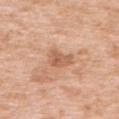  biopsy_status: not biopsied; imaged during a skin examination
  lighting: white-light
  patient:
    sex: female
    age_approx: 40
  lesion_size:
    long_diameter_mm_approx: 3.0
  image:
    source: total-body photography crop
    field_of_view_mm: 15
  site: upper back